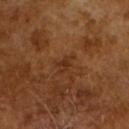The lesion was photographed on a routine skin check and not biopsied; there is no pathology result. A male patient, in their mid-60s. This image is a 15 mm lesion crop taken from a total-body photograph. Automated image analysis of the tile measured a lesion area of about 3 mm², an outline eccentricity of about 0.8 (0 = round, 1 = elongated), and a symmetry-axis asymmetry near 0.5. And it measured a border-irregularity rating of about 5.5/10, a color-variation rating of about 0.5/10, and radial color variation of about 0. The analysis additionally found an automated nevus-likeness rating near 0 out of 100 and a lesion-detection confidence of about 100/100. The tile uses cross-polarized illumination. About 2.5 mm across.